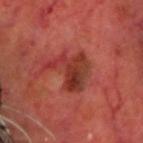Impression: The lesion was photographed on a routine skin check and not biopsied; there is no pathology result. Acquisition and patient details: From the head or neck. The lesion-visualizer software estimated an eccentricity of roughly 0.65 and two-axis asymmetry of about 0.6. And it measured a mean CIELAB color near L≈36 a*≈31 b*≈29, roughly 9 lightness units darker than nearby skin, and a normalized lesion–skin contrast near 8. And it measured border irregularity of about 8 on a 0–10 scale. The analysis additionally found a nevus-likeness score of about 0/100 and lesion-presence confidence of about 100/100. Captured under cross-polarized illumination. A male subject aged 68 to 72. A lesion tile, about 15 mm wide, cut from a 3D total-body photograph. Measured at roughly 5 mm in maximum diameter.